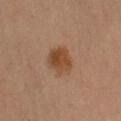Q: What did automated image analysis measure?
A: a footprint of about 9.5 mm², a shape eccentricity near 0.55, and two-axis asymmetry of about 0.15; internal color variation of about 4.5 on a 0–10 scale and radial color variation of about 1.5
Q: What is the imaging modality?
A: ~15 mm crop, total-body skin-cancer survey
Q: Where on the body is the lesion?
A: the left upper arm
Q: What lighting was used for the tile?
A: cross-polarized
Q: Who is the patient?
A: male, in their mid- to late 50s